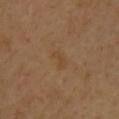Q: Was this lesion biopsied?
A: catalogued during a skin exam; not biopsied
Q: Patient demographics?
A: male, roughly 40 years of age
Q: What is the anatomic site?
A: the chest
Q: How was the tile lit?
A: cross-polarized
Q: What is the imaging modality?
A: total-body-photography crop, ~15 mm field of view